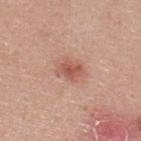No biopsy was performed on this lesion — it was imaged during a full skin examination and was not determined to be concerning.
This image is a 15 mm lesion crop taken from a total-body photograph.
A male subject in their mid- to late 20s.
Measured at roughly 3 mm in maximum diameter.
On the upper back.
This is a white-light tile.